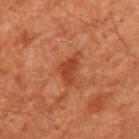Imaged during a routine full-body skin examination; the lesion was not biopsied and no histopathology is available.
Imaged with cross-polarized lighting.
A 15 mm close-up tile from a total-body photography series done for melanoma screening.
The lesion is located on the left upper arm.
A male patient in their 60s.
Automated tile analysis of the lesion measured a border-irregularity rating of about 4.5/10, a color-variation rating of about 1.5/10, and radial color variation of about 0.5. The software also gave a classifier nevus-likeness of about 20/100 and lesion-presence confidence of about 100/100.
Measured at roughly 4 mm in maximum diameter.